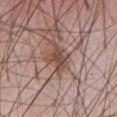Assessment: Recorded during total-body skin imaging; not selected for excision or biopsy. Background: A male patient, aged 58–62. The recorded lesion diameter is about 3.5 mm. A 15 mm close-up tile from a total-body photography series done for melanoma screening. The lesion is located on the chest. An algorithmic analysis of the crop reported a lesion area of about 5.5 mm², an eccentricity of roughly 0.75, and a symmetry-axis asymmetry near 0.35. And it measured a classifier nevus-likeness of about 5/100 and lesion-presence confidence of about 90/100.